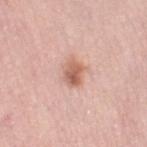Assessment: Imaged during a routine full-body skin examination; the lesion was not biopsied and no histopathology is available. Clinical summary: Cropped from a whole-body photographic skin survey; the tile spans about 15 mm. A female patient in their mid- to late 60s. The tile uses white-light illumination. From the right thigh. The lesion-visualizer software estimated a lesion–skin lightness drop of about 12 and a normalized lesion–skin contrast near 8. The analysis additionally found border irregularity of about 2 on a 0–10 scale, a color-variation rating of about 5.5/10, and radial color variation of about 2. The software also gave lesion-presence confidence of about 100/100. About 3 mm across.